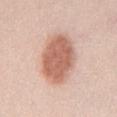The lesion was photographed on a routine skin check and not biopsied; there is no pathology result. The patient is a male approximately 35 years of age. A lesion tile, about 15 mm wide, cut from a 3D total-body photograph. Located on the front of the torso.